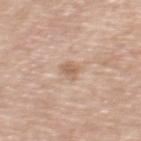notes: total-body-photography surveillance lesion; no biopsy | body site: the upper back | illumination: white-light illumination | acquisition: ~15 mm crop, total-body skin-cancer survey | subject: female, aged 53 to 57 | diameter: about 2.5 mm.